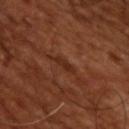Q: Was this lesion biopsied?
A: no biopsy performed (imaged during a skin exam)
Q: How was this image acquired?
A: ~15 mm crop, total-body skin-cancer survey
Q: How was the tile lit?
A: cross-polarized
Q: Patient demographics?
A: male, about 65 years old